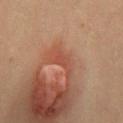Notes:
• notes — no biopsy performed (imaged during a skin exam)
• body site — the mid back
• illumination — cross-polarized illumination
• image source — ~15 mm crop, total-body skin-cancer survey
• patient — female, roughly 35 years of age